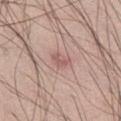Assessment:
Part of a total-body skin-imaging series; this lesion was reviewed on a skin check and was not flagged for biopsy.
Image and clinical context:
Located on the left thigh. A roughly 15 mm field-of-view crop from a total-body skin photograph. The tile uses white-light illumination. A male patient, aged 48–52. An algorithmic analysis of the crop reported a border-irregularity rating of about 3/10, a within-lesion color-variation index near 3.5/10, and radial color variation of about 1.5.